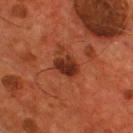Impression: Imaged during a routine full-body skin examination; the lesion was not biopsied and no histopathology is available. Context: The lesion is located on the chest. A male patient aged 53–57. A 15 mm crop from a total-body photograph taken for skin-cancer surveillance.